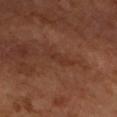Q: Was a biopsy performed?
A: total-body-photography surveillance lesion; no biopsy
Q: How was this image acquired?
A: 15 mm crop, total-body photography
Q: Patient demographics?
A: male, about 65 years old
Q: What lighting was used for the tile?
A: cross-polarized illumination